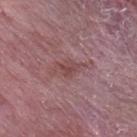Part of a total-body skin-imaging series; this lesion was reviewed on a skin check and was not flagged for biopsy.
A 15 mm close-up tile from a total-body photography series done for melanoma screening.
About 4 mm across.
The lesion is on the back.
The subject is a male in their mid- to late 50s.
Automated image analysis of the tile measured a lesion area of about 5.5 mm², an outline eccentricity of about 0.85 (0 = round, 1 = elongated), and two-axis asymmetry of about 0.3. The analysis additionally found a border-irregularity index near 4.5/10 and a within-lesion color-variation index near 2.5/10. And it measured a classifier nevus-likeness of about 0/100 and a detector confidence of about 90 out of 100 that the crop contains a lesion.